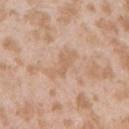workup: total-body-photography surveillance lesion; no biopsy
subject: female, approximately 25 years of age
anatomic site: the right upper arm
lighting: white-light illumination
image: ~15 mm tile from a whole-body skin photo